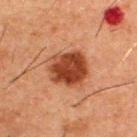Notes:
• workup · no biopsy performed (imaged during a skin exam)
• site · the upper back
• imaging modality · 15 mm crop, total-body photography
• subject · male, aged 48–52